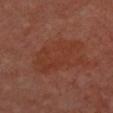Case summary:
– workup · catalogued during a skin exam; not biopsied
– acquisition · total-body-photography crop, ~15 mm field of view
– diameter · ≈7.5 mm
– patient · female, aged around 50
– location · the chest